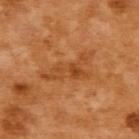- follow-up · no biopsy performed (imaged during a skin exam)
- automated metrics · an area of roughly 11 mm² and a shape-asymmetry score of about 0.55 (0 = symmetric); an average lesion color of about L≈49 a*≈27 b*≈43 (CIELAB) and a lesion–skin lightness drop of about 8
- patient · female, in their mid- to late 50s
- tile lighting · cross-polarized illumination
- location · the upper back
- image · total-body-photography crop, ~15 mm field of view